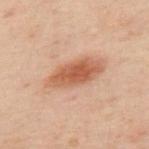  biopsy_status: not biopsied; imaged during a skin examination
  lesion_size:
    long_diameter_mm_approx: 7.0
  patient:
    sex: male
    age_approx: 50
  site: mid back
  lighting: cross-polarized
  image:
    source: total-body photography crop
    field_of_view_mm: 15